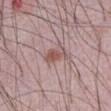Q: Is there a histopathology result?
A: catalogued during a skin exam; not biopsied
Q: How large is the lesion?
A: ~3.5 mm (longest diameter)
Q: What kind of image is this?
A: 15 mm crop, total-body photography
Q: How was the tile lit?
A: white-light
Q: What is the anatomic site?
A: the front of the torso
Q: What are the patient's age and sex?
A: male, about 65 years old